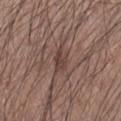Findings:
- notes — imaged on a skin check; not biopsied
- anatomic site — the left forearm
- acquisition — ~15 mm crop, total-body skin-cancer survey
- patient — male, approximately 55 years of age
- tile lighting — white-light
- size — about 3 mm